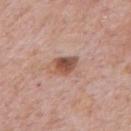Clinical summary:
A region of skin cropped from a whole-body photographic capture, roughly 15 mm wide. The recorded lesion diameter is about 3 mm. A male patient, in their 80s. The tile uses white-light illumination. The lesion is on the chest.